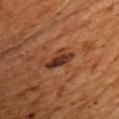  biopsy_status: not biopsied; imaged during a skin examination